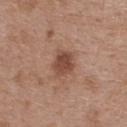Notes:
* follow-up: total-body-photography surveillance lesion; no biopsy
* lesion size: ~3 mm (longest diameter)
* image source: 15 mm crop, total-body photography
* patient: female, in their 40s
* anatomic site: the upper back